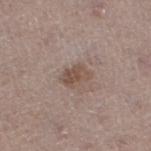Captured during whole-body skin photography for melanoma surveillance; the lesion was not biopsied. Captured under white-light illumination. The subject is a female in their 50s. Cropped from a total-body skin-imaging series; the visible field is about 15 mm. On the leg. Automated tile analysis of the lesion measured an area of roughly 4 mm², an outline eccentricity of about 0.7 (0 = round, 1 = elongated), and two-axis asymmetry of about 0.3. It also reported an average lesion color of about L≈49 a*≈16 b*≈24 (CIELAB) and a lesion-to-skin contrast of about 7.5 (normalized; higher = more distinct). The analysis additionally found a color-variation rating of about 2.5/10 and a peripheral color-asymmetry measure near 1. And it measured a classifier nevus-likeness of about 15/100 and a detector confidence of about 100 out of 100 that the crop contains a lesion. The lesion's longest dimension is about 3 mm.